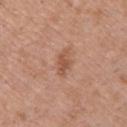follow-up = imaged on a skin check; not biopsied
location = the upper back
image source = ~15 mm crop, total-body skin-cancer survey
patient = male, approximately 50 years of age
illumination = white-light